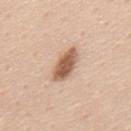Impression:
The lesion was photographed on a routine skin check and not biopsied; there is no pathology result.
Acquisition and patient details:
Approximately 4.5 mm at its widest. A male subject aged around 45. The lesion is located on the mid back. Captured under white-light illumination. Cropped from a total-body skin-imaging series; the visible field is about 15 mm.